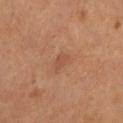| field | value |
|---|---|
| workup | no biopsy performed (imaged during a skin exam) |
| image | 15 mm crop, total-body photography |
| subject | male, in their mid-50s |
| location | the leg |
| tile lighting | cross-polarized |
| diameter | about 2.5 mm |
| TBP lesion metrics | a shape eccentricity near 0.8 and a symmetry-axis asymmetry near 0.35 |